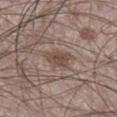Findings:
– notes — catalogued during a skin exam; not biopsied
– acquisition — 15 mm crop, total-body photography
– patient — male, aged around 60
– location — the right lower leg
– TBP lesion metrics — a lesion color around L≈47 a*≈16 b*≈22 in CIELAB, a lesion–skin lightness drop of about 8, and a normalized lesion–skin contrast near 6.5; a border-irregularity rating of about 3/10 and radial color variation of about 1
– lesion diameter — ~3.5 mm (longest diameter)